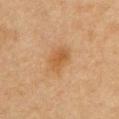Impression: Part of a total-body skin-imaging series; this lesion was reviewed on a skin check and was not flagged for biopsy. Clinical summary: Automated tile analysis of the lesion measured a mean CIELAB color near L≈48 a*≈19 b*≈36, about 7 CIELAB-L* units darker than the surrounding skin, and a normalized border contrast of about 6.5. And it measured a nevus-likeness score of about 50/100 and a detector confidence of about 100 out of 100 that the crop contains a lesion. A female patient, aged 38 to 42. About 3 mm across. The lesion is on the chest. Cropped from a total-body skin-imaging series; the visible field is about 15 mm. Imaged with cross-polarized lighting.